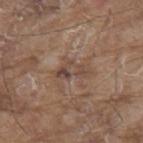| feature | finding |
|---|---|
| notes | no biopsy performed (imaged during a skin exam) |
| image-analysis metrics | a footprint of about 5.5 mm² and a shape eccentricity near 0.9; a lesion color around L≈45 a*≈16 b*≈24 in CIELAB and a lesion-to-skin contrast of about 6.5 (normalized; higher = more distinct); a nevus-likeness score of about 0/100 and lesion-presence confidence of about 95/100 |
| subject | male, roughly 80 years of age |
| illumination | white-light |
| lesion diameter | ≈4 mm |
| site | the mid back |
| image source | total-body-photography crop, ~15 mm field of view |